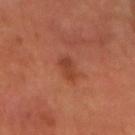{
  "biopsy_status": "not biopsied; imaged during a skin examination",
  "site": "head or neck",
  "lesion_size": {
    "long_diameter_mm_approx": 2.5
  },
  "patient": {
    "sex": "male",
    "age_approx": 55
  },
  "image": {
    "source": "total-body photography crop",
    "field_of_view_mm": 15
  }
}